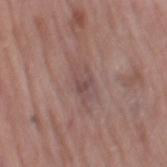{"site": "leg", "patient": {"sex": "female", "age_approx": 70}, "automated_metrics": {"area_mm2_approx": 7.0, "eccentricity": 0.9, "shape_asymmetry": 0.35, "border_irregularity_0_10": 4.0, "color_variation_0_10": 3.5, "peripheral_color_asymmetry": 1.0, "nevus_likeness_0_100": 0, "lesion_detection_confidence_0_100": 80}, "image": {"source": "total-body photography crop", "field_of_view_mm": 15}}